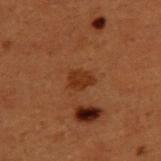A male subject in their 50s.
The recorded lesion diameter is about 3 mm.
A roughly 15 mm field-of-view crop from a total-body skin photograph.
The lesion is on the upper back.
Captured under cross-polarized illumination.
The total-body-photography lesion software estimated a lesion area of about 4.5 mm², an eccentricity of roughly 0.65, and two-axis asymmetry of about 0.3. And it measured an automated nevus-likeness rating near 60 out of 100 and a detector confidence of about 100 out of 100 that the crop contains a lesion.